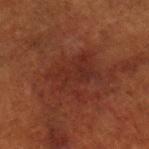Recorded during total-body skin imaging; not selected for excision or biopsy. From the left lower leg. The lesion-visualizer software estimated a border-irregularity rating of about 5.5/10 and a peripheral color-asymmetry measure near 1. It also reported a nevus-likeness score of about 0/100. A 15 mm close-up tile from a total-body photography series done for melanoma screening. A female patient, approximately 80 years of age. Longest diameter approximately 5.5 mm.